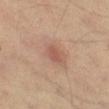Q: Was this lesion biopsied?
A: total-body-photography surveillance lesion; no biopsy
Q: How was the tile lit?
A: cross-polarized
Q: What are the patient's age and sex?
A: male, roughly 55 years of age
Q: What is the imaging modality?
A: total-body-photography crop, ~15 mm field of view
Q: Where on the body is the lesion?
A: the left thigh
Q: What did automated image analysis measure?
A: a lesion area of about 3 mm² and a shape eccentricity near 0.8; an automated nevus-likeness rating near 50 out of 100 and a lesion-detection confidence of about 100/100